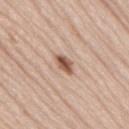Impression:
No biopsy was performed on this lesion — it was imaged during a full skin examination and was not determined to be concerning.
Clinical summary:
Approximately 3 mm at its widest. A 15 mm close-up extracted from a 3D total-body photography capture. A male patient, aged around 75. Automated tile analysis of the lesion measured a lesion-detection confidence of about 100/100. Captured under white-light illumination. On the back.